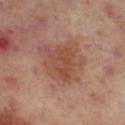Notes:
- notes: catalogued during a skin exam; not biopsied
- subject: female, in their mid- to late 50s
- image: total-body-photography crop, ~15 mm field of view
- TBP lesion metrics: a border-irregularity rating of about 3.5/10, a within-lesion color-variation index near 3/10, and a peripheral color-asymmetry measure near 1; an automated nevus-likeness rating near 0 out of 100 and lesion-presence confidence of about 100/100
- size: ~4.5 mm (longest diameter)
- location: the right lower leg